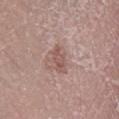The lesion is on the left lower leg.
A male patient aged 43 to 47.
About 3 mm across.
Automated tile analysis of the lesion measured an area of roughly 3.5 mm² and two-axis asymmetry of about 0.25. It also reported border irregularity of about 3 on a 0–10 scale. And it measured a classifier nevus-likeness of about 0/100 and a detector confidence of about 100 out of 100 that the crop contains a lesion.
A close-up tile cropped from a whole-body skin photograph, about 15 mm across.
The tile uses white-light illumination.